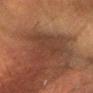<record>
  <biopsy_status>not biopsied; imaged during a skin examination</biopsy_status>
  <site>head or neck</site>
  <patient>
    <sex>male</sex>
    <age_approx>60</age_approx>
  </patient>
  <image>
    <source>total-body photography crop</source>
    <field_of_view_mm>15</field_of_view_mm>
  </image>
</record>